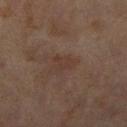anatomic site=the right lower leg | image=~15 mm crop, total-body skin-cancer survey | lesion size=~3 mm (longest diameter) | TBP lesion metrics=a border-irregularity rating of about 5/10 and internal color variation of about 1 on a 0–10 scale; an automated nevus-likeness rating near 0 out of 100 | lighting=cross-polarized | patient=female, roughly 60 years of age.